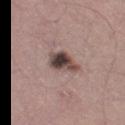Q: Is there a histopathology result?
A: imaged on a skin check; not biopsied
Q: What lighting was used for the tile?
A: white-light
Q: What is the lesion's diameter?
A: ≈4 mm
Q: How was this image acquired?
A: ~15 mm crop, total-body skin-cancer survey
Q: What is the anatomic site?
A: the left thigh
Q: Patient demographics?
A: male, roughly 60 years of age